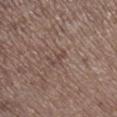This lesion was catalogued during total-body skin photography and was not selected for biopsy.
Cropped from a whole-body photographic skin survey; the tile spans about 15 mm.
From the right lower leg.
This is a white-light tile.
An algorithmic analysis of the crop reported an outline eccentricity of about 0.95 (0 = round, 1 = elongated). And it measured an average lesion color of about L≈45 a*≈17 b*≈22 (CIELAB) and about 6 CIELAB-L* units darker than the surrounding skin.
Measured at roughly 2.5 mm in maximum diameter.
The subject is a male about 70 years old.